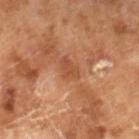The recorded lesion diameter is about 2.5 mm. A male patient aged approximately 65. The total-body-photography lesion software estimated a lesion area of about 4 mm² and a symmetry-axis asymmetry near 0.3. It also reported border irregularity of about 3 on a 0–10 scale and a color-variation rating of about 2.5/10. Imaged with cross-polarized lighting. A region of skin cropped from a whole-body photographic capture, roughly 15 mm wide.